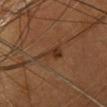Clinical impression: The lesion was tiled from a total-body skin photograph and was not biopsied. Context: A 15 mm close-up extracted from a 3D total-body photography capture. The lesion's longest dimension is about 3 mm. Imaged with cross-polarized lighting. The lesion is located on the head or neck. The lesion-visualizer software estimated a footprint of about 4.5 mm², an outline eccentricity of about 0.75 (0 = round, 1 = elongated), and a shape-asymmetry score of about 0.5 (0 = symmetric). And it measured a border-irregularity rating of about 5/10, a within-lesion color-variation index near 3/10, and a peripheral color-asymmetry measure near 1. The analysis additionally found a detector confidence of about 100 out of 100 that the crop contains a lesion. The subject is a female aged 33 to 37.